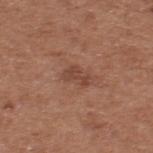follow-up = catalogued during a skin exam; not biopsied
image source = ~15 mm tile from a whole-body skin photo
subject = male, in their mid- to late 50s
anatomic site = the upper back
automated lesion analysis = a border-irregularity rating of about 4.5/10, a color-variation rating of about 1.5/10, and a peripheral color-asymmetry measure near 0.5